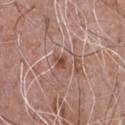| field | value |
|---|---|
| notes | catalogued during a skin exam; not biopsied |
| lesion diameter | ~3 mm (longest diameter) |
| location | the chest |
| image | total-body-photography crop, ~15 mm field of view |
| illumination | white-light |
| subject | male, roughly 70 years of age |
| automated lesion analysis | a footprint of about 4 mm², an outline eccentricity of about 0.8 (0 = round, 1 = elongated), and two-axis asymmetry of about 0.25 |